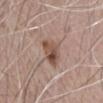The lesion is on the head or neck. About 4 mm across. A male subject aged approximately 70. A 15 mm close-up extracted from a 3D total-body photography capture.